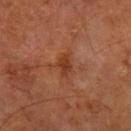patient=male, aged 63–67
site=the leg
image=total-body-photography crop, ~15 mm field of view